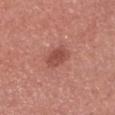Assessment:
No biopsy was performed on this lesion — it was imaged during a full skin examination and was not determined to be concerning.
Acquisition and patient details:
A region of skin cropped from a whole-body photographic capture, roughly 15 mm wide. A male patient in their 30s. About 2.5 mm across. The tile uses white-light illumination. Automated image analysis of the tile measured a lesion area of about 5 mm², an outline eccentricity of about 0.3 (0 = round, 1 = elongated), and a symmetry-axis asymmetry near 0.15. And it measured a lesion color around L≈49 a*≈28 b*≈27 in CIELAB, roughly 10 lightness units darker than nearby skin, and a lesion-to-skin contrast of about 7 (normalized; higher = more distinct). From the head or neck.